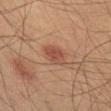Q: Is there a histopathology result?
A: imaged on a skin check; not biopsied
Q: What did automated image analysis measure?
A: a footprint of about 5.5 mm²; a mean CIELAB color near L≈44 a*≈22 b*≈28 and about 9 CIELAB-L* units darker than the surrounding skin; a within-lesion color-variation index near 3/10 and peripheral color asymmetry of about 1
Q: How was the tile lit?
A: cross-polarized illumination
Q: Patient demographics?
A: male, aged around 60
Q: Lesion location?
A: the lower back
Q: What is the lesion's diameter?
A: ~3.5 mm (longest diameter)
Q: What kind of image is this?
A: ~15 mm crop, total-body skin-cancer survey